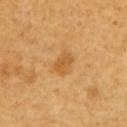Impression:
The lesion was tiled from a total-body skin photograph and was not biopsied.
Acquisition and patient details:
An algorithmic analysis of the crop reported an area of roughly 4 mm², an eccentricity of roughly 0.75, and two-axis asymmetry of about 0.25. The analysis additionally found peripheral color asymmetry of about 1.5. It also reported a classifier nevus-likeness of about 60/100 and lesion-presence confidence of about 100/100. The subject is a female aged 53 to 57. A region of skin cropped from a whole-body photographic capture, roughly 15 mm wide. On the chest. Approximately 2.5 mm at its widest.